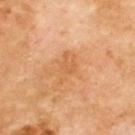This lesion was catalogued during total-body skin photography and was not selected for biopsy. Automated image analysis of the tile measured a classifier nevus-likeness of about 0/100 and a lesion-detection confidence of about 100/100. Captured under cross-polarized illumination. A 15 mm close-up tile from a total-body photography series done for melanoma screening. A male patient, aged around 70. The lesion is on the upper back.